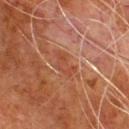workup=imaged on a skin check; not biopsied | body site=the chest | illumination=cross-polarized illumination | subject=male, roughly 80 years of age | diameter=≈3.5 mm | image=total-body-photography crop, ~15 mm field of view.